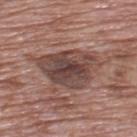Recorded during total-body skin imaging; not selected for excision or biopsy. The lesion's longest dimension is about 7.5 mm. A lesion tile, about 15 mm wide, cut from a 3D total-body photograph. Located on the upper back. A male patient in their 70s.